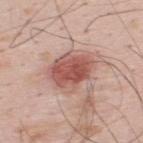Q: Is there a histopathology result?
A: catalogued during a skin exam; not biopsied
Q: Who is the patient?
A: male, in their mid-30s
Q: How was this image acquired?
A: ~15 mm crop, total-body skin-cancer survey
Q: What is the lesion's diameter?
A: ≈5 mm
Q: Where on the body is the lesion?
A: the upper back